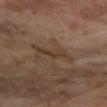Q: Is there a histopathology result?
A: imaged on a skin check; not biopsied
Q: What kind of image is this?
A: total-body-photography crop, ~15 mm field of view
Q: What lighting was used for the tile?
A: cross-polarized
Q: What is the anatomic site?
A: the right forearm
Q: Who is the patient?
A: female, roughly 45 years of age
Q: Lesion size?
A: ~4.5 mm (longest diameter)
Q: What did automated image analysis measure?
A: a mean CIELAB color near L≈38 a*≈16 b*≈26, a lesion–skin lightness drop of about 6, and a lesion-to-skin contrast of about 6 (normalized; higher = more distinct); internal color variation of about 3 on a 0–10 scale; a classifier nevus-likeness of about 0/100 and lesion-presence confidence of about 65/100